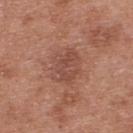On the upper back. A male patient aged 28–32. The tile uses white-light illumination. This image is a 15 mm lesion crop taken from a total-body photograph.